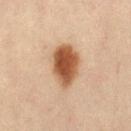Recorded during total-body skin imaging; not selected for excision or biopsy. The subject is a female aged 43–47. A 15 mm crop from a total-body photograph taken for skin-cancer surveillance. The total-body-photography lesion software estimated a lesion area of about 13 mm², a shape eccentricity near 0.7, and two-axis asymmetry of about 0.2. And it measured a mean CIELAB color near L≈46 a*≈20 b*≈31 and a normalized border contrast of about 11.5. The software also gave a border-irregularity index near 2/10 and peripheral color asymmetry of about 1.5. And it measured a nevus-likeness score of about 100/100 and lesion-presence confidence of about 100/100. The lesion is on the left thigh. Approximately 5 mm at its widest.